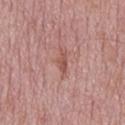On the mid back.
A male patient, in their mid- to late 70s.
A roughly 15 mm field-of-view crop from a total-body skin photograph.
Measured at roughly 3.5 mm in maximum diameter.